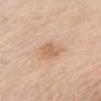follow-up=catalogued during a skin exam; not biopsied | anatomic site=the front of the torso | imaging modality=~15 mm tile from a whole-body skin photo | lesion size=~3 mm (longest diameter) | subject=female, aged 58 to 62 | lighting=white-light illumination.